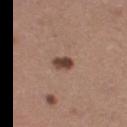{"image": {"source": "total-body photography crop", "field_of_view_mm": 15}, "patient": {"sex": "female", "age_approx": 35}, "site": "right thigh", "lighting": "white-light", "lesion_size": {"long_diameter_mm_approx": 2.5}, "automated_metrics": {"area_mm2_approx": 3.5, "eccentricity": 0.8, "shape_asymmetry": 0.2, "cielab_L": 43, "cielab_a": 19, "cielab_b": 24, "vs_skin_darker_L": 15.0, "vs_skin_contrast_norm": 11.0}}